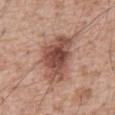follow-up = total-body-photography surveillance lesion; no biopsy
image = 15 mm crop, total-body photography
TBP lesion metrics = an average lesion color of about L≈49 a*≈22 b*≈26 (CIELAB), about 14 CIELAB-L* units darker than the surrounding skin, and a normalized border contrast of about 9.5; a peripheral color-asymmetry measure near 2
diameter = ≈6 mm
lighting = white-light
anatomic site = the front of the torso
subject = male, approximately 60 years of age